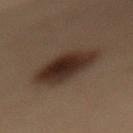The lesion was photographed on a routine skin check and not biopsied; there is no pathology result. The patient is a female roughly 50 years of age. Located on the mid back. This image is a 15 mm lesion crop taken from a total-body photograph.